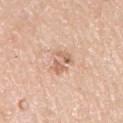Acquisition and patient details: Captured under white-light illumination. Approximately 3 mm at its widest. On the right upper arm. The total-body-photography lesion software estimated an eccentricity of roughly 0.6. The analysis additionally found a border-irregularity rating of about 6/10, a within-lesion color-variation index near 2.5/10, and a peripheral color-asymmetry measure near 0.5. It also reported lesion-presence confidence of about 100/100. The subject is a male in their 60s. Cropped from a whole-body photographic skin survey; the tile spans about 15 mm.